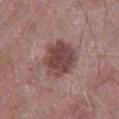Background: A male patient, approximately 55 years of age. The total-body-photography lesion software estimated a border-irregularity index near 2/10, a color-variation rating of about 4.5/10, and peripheral color asymmetry of about 1.5. And it measured a nevus-likeness score of about 10/100. About 5 mm across. A 15 mm close-up tile from a total-body photography series done for melanoma screening. On the leg.